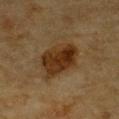Q: Was this lesion biopsied?
A: imaged on a skin check; not biopsied
Q: What kind of image is this?
A: total-body-photography crop, ~15 mm field of view
Q: How large is the lesion?
A: ≈5.5 mm
Q: How was the tile lit?
A: cross-polarized
Q: Patient demographics?
A: male, roughly 85 years of age or older
Q: Where on the body is the lesion?
A: the front of the torso
Q: Automated lesion metrics?
A: an average lesion color of about L≈27 a*≈16 b*≈28 (CIELAB), about 10 CIELAB-L* units darker than the surrounding skin, and a lesion-to-skin contrast of about 11 (normalized; higher = more distinct)